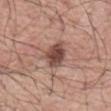biopsy status = catalogued during a skin exam; not biopsied | image = total-body-photography crop, ~15 mm field of view | site = the mid back | subject = male, aged 53 to 57.